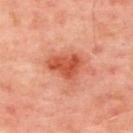Findings:
- lesion diameter · ~4 mm (longest diameter)
- subject · male, roughly 50 years of age
- illumination · cross-polarized
- image · 15 mm crop, total-body photography
- site · the upper back